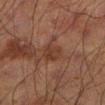Findings:
- follow-up: catalogued during a skin exam; not biopsied
- lighting: cross-polarized illumination
- anatomic site: the left lower leg
- subject: male, approximately 70 years of age
- size: ~3 mm (longest diameter)
- image source: ~15 mm crop, total-body skin-cancer survey
- image-analysis metrics: a shape eccentricity near 0.65; a color-variation rating of about 2/10 and a peripheral color-asymmetry measure near 1; a nevus-likeness score of about 0/100 and lesion-presence confidence of about 100/100